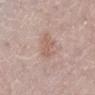Recorded during total-body skin imaging; not selected for excision or biopsy. This image is a 15 mm lesion crop taken from a total-body photograph. About 2.5 mm across. The tile uses white-light illumination. A female subject, aged approximately 55. Located on the leg.